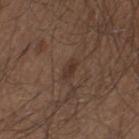- biopsy status: catalogued during a skin exam; not biopsied
- image-analysis metrics: an automated nevus-likeness rating near 20 out of 100
- imaging modality: 15 mm crop, total-body photography
- patient: male, aged around 45
- illumination: white-light illumination
- lesion diameter: ~2.5 mm (longest diameter)
- body site: the left thigh